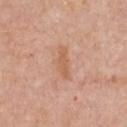Impression: Recorded during total-body skin imaging; not selected for excision or biopsy. Background: Captured under white-light illumination. The total-body-photography lesion software estimated a lesion area of about 4.5 mm², a shape eccentricity near 0.95, and two-axis asymmetry of about 0.4. And it measured a classifier nevus-likeness of about 0/100 and lesion-presence confidence of about 100/100. A 15 mm close-up extracted from a 3D total-body photography capture. A female subject aged 68–72. From the chest.